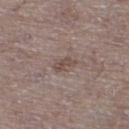workup: catalogued during a skin exam; not biopsied | body site: the leg | acquisition: 15 mm crop, total-body photography | patient: male, in their mid-60s.